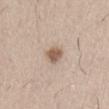Recorded during total-body skin imaging; not selected for excision or biopsy. On the lower back. The lesion's longest dimension is about 2.5 mm. Captured under white-light illumination. The patient is a male in their 60s. Cropped from a whole-body photographic skin survey; the tile spans about 15 mm. The total-body-photography lesion software estimated a border-irregularity rating of about 2/10, a within-lesion color-variation index near 2.5/10, and a peripheral color-asymmetry measure near 1.